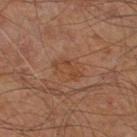{"biopsy_status": "not biopsied; imaged during a skin examination", "site": "right leg", "lighting": "cross-polarized", "patient": {"sex": "male", "age_approx": 60}, "image": {"source": "total-body photography crop", "field_of_view_mm": 15}, "lesion_size": {"long_diameter_mm_approx": 3.5}}